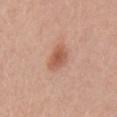biopsy status: total-body-photography surveillance lesion; no biopsy | lesion diameter: about 3 mm | subject: female, in their 40s | site: the left upper arm | automated metrics: a footprint of about 6.5 mm², an eccentricity of roughly 0.5, and a symmetry-axis asymmetry near 0.25; a classifier nevus-likeness of about 95/100 and a detector confidence of about 100 out of 100 that the crop contains a lesion | image: ~15 mm crop, total-body skin-cancer survey.